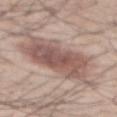Part of a total-body skin-imaging series; this lesion was reviewed on a skin check and was not flagged for biopsy. Imaged with white-light lighting. The subject is a male about 55 years old. Longest diameter approximately 9.5 mm. A close-up tile cropped from a whole-body skin photograph, about 15 mm across. The lesion is on the back. An algorithmic analysis of the crop reported an automated nevus-likeness rating near 85 out of 100 and lesion-presence confidence of about 100/100.